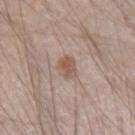<lesion>
<biopsy_status>not biopsied; imaged during a skin examination</biopsy_status>
<lesion_size>
  <long_diameter_mm_approx>3.0</long_diameter_mm_approx>
</lesion_size>
<image>
  <source>total-body photography crop</source>
  <field_of_view_mm>15</field_of_view_mm>
</image>
<lighting>white-light</lighting>
<automated_metrics>
  <area_mm2_approx>5.0</area_mm2_approx>
  <eccentricity>0.55</eccentricity>
  <shape_asymmetry>0.25</shape_asymmetry>
  <nevus_likeness_0_100>85</nevus_likeness_0_100>
  <lesion_detection_confidence_0_100>100</lesion_detection_confidence_0_100>
</automated_metrics>
<site>right forearm</site>
<patient>
  <sex>male</sex>
  <age_approx>35</age_approx>
</patient>
</lesion>